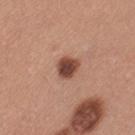<case>
<biopsy_status>not biopsied; imaged during a skin examination</biopsy_status>
<patient>
  <sex>female</sex>
  <age_approx>35</age_approx>
</patient>
<automated_metrics>
  <eccentricity>0.4</eccentricity>
  <shape_asymmetry>0.2</shape_asymmetry>
  <nevus_likeness_0_100>95</nevus_likeness_0_100>
  <lesion_detection_confidence_0_100>100</lesion_detection_confidence_0_100>
</automated_metrics>
<site>leg</site>
<lesion_size>
  <long_diameter_mm_approx>2.5</long_diameter_mm_approx>
</lesion_size>
<image>
  <source>total-body photography crop</source>
  <field_of_view_mm>15</field_of_view_mm>
</image>
</case>